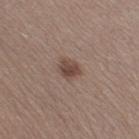{
  "lighting": "white-light",
  "image": {
    "source": "total-body photography crop",
    "field_of_view_mm": 15
  },
  "site": "right thigh",
  "lesion_size": {
    "long_diameter_mm_approx": 2.5
  },
  "patient": {
    "sex": "female",
    "age_approx": 40
  }
}